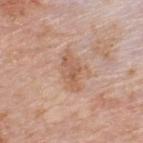Q: Was a biopsy performed?
A: imaged on a skin check; not biopsied
Q: Where on the body is the lesion?
A: the upper back
Q: Illumination type?
A: white-light illumination
Q: How large is the lesion?
A: ~4.5 mm (longest diameter)
Q: What is the imaging modality?
A: ~15 mm crop, total-body skin-cancer survey
Q: Who is the patient?
A: male, in their mid-70s